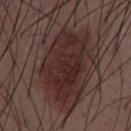Impression:
Imaged during a routine full-body skin examination; the lesion was not biopsied and no histopathology is available.
Acquisition and patient details:
The total-body-photography lesion software estimated a nevus-likeness score of about 95/100 and a detector confidence of about 100 out of 100 that the crop contains a lesion. The lesion is located on the abdomen. Longest diameter approximately 9.5 mm. The subject is a male in their 50s. The tile uses white-light illumination. Cropped from a total-body skin-imaging series; the visible field is about 15 mm.